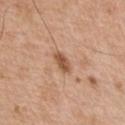Acquisition and patient details: This image is a 15 mm lesion crop taken from a total-body photograph. A male subject in their mid- to late 50s. Located on the chest. Imaged with white-light lighting.